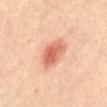workup: total-body-photography surveillance lesion; no biopsy | imaging modality: total-body-photography crop, ~15 mm field of view | TBP lesion metrics: a lesion area of about 9 mm², an outline eccentricity of about 0.7 (0 = round, 1 = elongated), and two-axis asymmetry of about 0.2; a border-irregularity index near 2/10, a within-lesion color-variation index near 4.5/10, and peripheral color asymmetry of about 1.5 | size: about 4 mm | tile lighting: cross-polarized | subject: female, approximately 65 years of age | body site: the front of the torso.